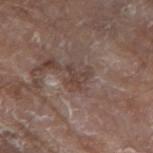Case summary:
– image source: total-body-photography crop, ~15 mm field of view
– size: about 2.5 mm
– image-analysis metrics: border irregularity of about 6 on a 0–10 scale, a within-lesion color-variation index near 2.5/10, and a peripheral color-asymmetry measure near 1; a classifier nevus-likeness of about 0/100 and a detector confidence of about 90 out of 100 that the crop contains a lesion
– patient: male, aged 78 to 82
– illumination: white-light
– body site: the left forearm